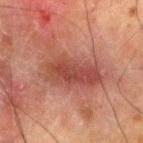Recorded during total-body skin imaging; not selected for excision or biopsy. The lesion is on the right thigh. Cropped from a whole-body photographic skin survey; the tile spans about 15 mm. A male subject aged 78–82.